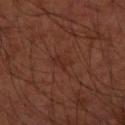{"biopsy_status": "not biopsied; imaged during a skin examination", "image": {"source": "total-body photography crop", "field_of_view_mm": 15}, "site": "left arm", "lighting": "cross-polarized", "patient": {"sex": "male", "age_approx": 50}}